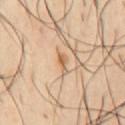Part of a total-body skin-imaging series; this lesion was reviewed on a skin check and was not flagged for biopsy. The lesion is on the chest. Captured under cross-polarized illumination. Cropped from a whole-body photographic skin survey; the tile spans about 15 mm. A male patient aged approximately 40.